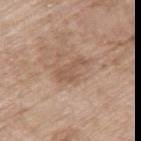Imaged during a routine full-body skin examination; the lesion was not biopsied and no histopathology is available. Imaged with white-light lighting. The lesion-visualizer software estimated a footprint of about 5 mm², an outline eccentricity of about 0.9 (0 = round, 1 = elongated), and a shape-asymmetry score of about 0.45 (0 = symmetric). It also reported a lesion color around L≈54 a*≈18 b*≈29 in CIELAB and roughly 8 lightness units darker than nearby skin. And it measured border irregularity of about 5.5 on a 0–10 scale and a peripheral color-asymmetry measure near 0.5. Cropped from a total-body skin-imaging series; the visible field is about 15 mm. A female subject aged around 75. The lesion is located on the back.